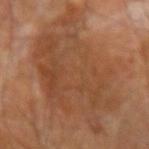biopsy status: total-body-photography surveillance lesion; no biopsy | tile lighting: cross-polarized illumination | imaging modality: ~15 mm crop, total-body skin-cancer survey | location: the right forearm | TBP lesion metrics: a footprint of about 70 mm² and an eccentricity of roughly 0.65; an average lesion color of about L≈44 a*≈21 b*≈32 (CIELAB), a lesion–skin lightness drop of about 7, and a normalized border contrast of about 6 | subject: male, aged 63–67.